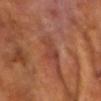Notes:
* biopsy status: no biopsy performed (imaged during a skin exam)
* diameter: ≈4 mm
* automated metrics: an area of roughly 6 mm² and two-axis asymmetry of about 0.45; a lesion–skin lightness drop of about 6 and a lesion-to-skin contrast of about 5.5 (normalized; higher = more distinct)
* anatomic site: the upper back
* patient: male, aged 58–62
* acquisition: ~15 mm crop, total-body skin-cancer survey
* illumination: cross-polarized